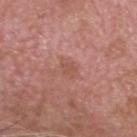The lesion was tiled from a total-body skin photograph and was not biopsied.
From the head or neck.
A male subject in their mid-70s.
A close-up tile cropped from a whole-body skin photograph, about 15 mm across.